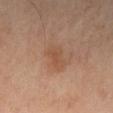Notes:
– workup · no biopsy performed (imaged during a skin exam)
– image-analysis metrics · an area of roughly 5 mm² and an outline eccentricity of about 0.8 (0 = round, 1 = elongated); border irregularity of about 2.5 on a 0–10 scale, internal color variation of about 1.5 on a 0–10 scale, and radial color variation of about 0.5
– lesion diameter · about 3 mm
– body site · the leg
– illumination · cross-polarized
– image · ~15 mm tile from a whole-body skin photo
– subject · male, aged around 65